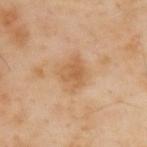Impression:
This lesion was catalogued during total-body skin photography and was not selected for biopsy.
Context:
A 15 mm close-up extracted from a 3D total-body photography capture. The tile uses cross-polarized illumination. The lesion is on the upper back. Approximately 3 mm at its widest. The subject is a male approximately 55 years of age.